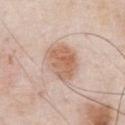Q: Is there a histopathology result?
A: catalogued during a skin exam; not biopsied
Q: What kind of image is this?
A: 15 mm crop, total-body photography
Q: What did automated image analysis measure?
A: a mean CIELAB color near L≈63 a*≈19 b*≈30, roughly 11 lightness units darker than nearby skin, and a normalized border contrast of about 8; border irregularity of about 2 on a 0–10 scale, internal color variation of about 5 on a 0–10 scale, and peripheral color asymmetry of about 1.5
Q: Who is the patient?
A: male, aged approximately 55
Q: How was the tile lit?
A: white-light illumination
Q: Lesion location?
A: the chest